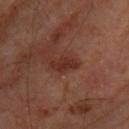patient:
  sex: male
  age_approx: 65
automated_metrics:
  area_mm2_approx: 4.5
  eccentricity: 0.9
  shape_asymmetry: 0.35
  cielab_L: 30
  cielab_a: 23
  cielab_b: 26
  vs_skin_darker_L: 8.0
  border_irregularity_0_10: 4.0
  color_variation_0_10: 1.0
  nevus_likeness_0_100: 0
image:
  source: total-body photography crop
  field_of_view_mm: 15
lesion_size:
  long_diameter_mm_approx: 3.5
lighting: cross-polarized
site: upper back